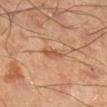Imaged during a routine full-body skin examination; the lesion was not biopsied and no histopathology is available. A male patient, aged approximately 45. Imaged with cross-polarized lighting. Approximately 3 mm at its widest. Located on the leg. A lesion tile, about 15 mm wide, cut from a 3D total-body photograph.